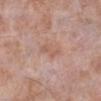This lesion was catalogued during total-body skin photography and was not selected for biopsy.
Cropped from a total-body skin-imaging series; the visible field is about 15 mm.
The lesion-visualizer software estimated an area of roughly 4 mm² and an eccentricity of roughly 0.9. The analysis additionally found an average lesion color of about L≈58 a*≈21 b*≈27 (CIELAB), roughly 7 lightness units darker than nearby skin, and a lesion-to-skin contrast of about 5 (normalized; higher = more distinct). It also reported a nevus-likeness score of about 0/100.
On the leg.
About 3.5 mm across.
The patient is a male approximately 75 years of age.